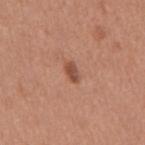Impression:
Imaged during a routine full-body skin examination; the lesion was not biopsied and no histopathology is available.
Background:
On the mid back. This is a white-light tile. Cropped from a total-body skin-imaging series; the visible field is about 15 mm. The recorded lesion diameter is about 2.5 mm. The patient is a male approximately 65 years of age.